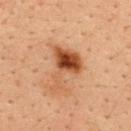Assessment: The lesion was tiled from a total-body skin photograph and was not biopsied. Acquisition and patient details: The patient is a male approximately 35 years of age. Measured at roughly 5.5 mm in maximum diameter. The lesion is located on the upper back. A 15 mm close-up extracted from a 3D total-body photography capture. Automated tile analysis of the lesion measured a lesion area of about 11 mm², an eccentricity of roughly 0.7, and a shape-asymmetry score of about 0.65 (0 = symmetric). The analysis additionally found a lesion color around L≈47 a*≈24 b*≈36 in CIELAB, roughly 14 lightness units darker than nearby skin, and a lesion-to-skin contrast of about 10.5 (normalized; higher = more distinct). The tile uses cross-polarized illumination.